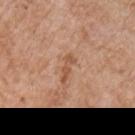notes=no biopsy performed (imaged during a skin exam)
image source=~15 mm crop, total-body skin-cancer survey
subject=male, aged 63 to 67
lesion diameter=about 3.5 mm
site=the chest
lighting=white-light